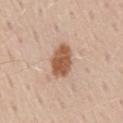This lesion was catalogued during total-body skin photography and was not selected for biopsy. A 15 mm close-up tile from a total-body photography series done for melanoma screening. A male patient aged 58 to 62. From the lower back. Measured at roughly 4 mm in maximum diameter.